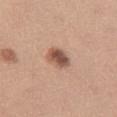Clinical summary:
From the left thigh. Approximately 3 mm at its widest. Imaged with white-light lighting. A female patient about 45 years old. Cropped from a whole-body photographic skin survey; the tile spans about 15 mm.